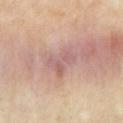Clinical impression: The lesion was tiled from a total-body skin photograph and was not biopsied. Context: Automated tile analysis of the lesion measured a footprint of about 7.5 mm², an outline eccentricity of about 0.7 (0 = round, 1 = elongated), and two-axis asymmetry of about 0.4. The analysis additionally found a border-irregularity rating of about 4.5/10 and a peripheral color-asymmetry measure near 1. On the arm. A female subject about 40 years old. Imaged with cross-polarized lighting. A close-up tile cropped from a whole-body skin photograph, about 15 mm across.